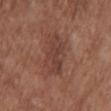biopsy status: imaged on a skin check; not biopsied
patient: female, roughly 75 years of age
acquisition: 15 mm crop, total-body photography
size: about 5.5 mm
site: the upper back